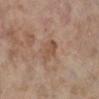- notes — total-body-photography surveillance lesion; no biopsy
- body site — the right lower leg
- image — total-body-photography crop, ~15 mm field of view
- subject — female, in their mid-80s or older
- size — ~3 mm (longest diameter)
- automated metrics — a lesion area of about 5 mm² and two-axis asymmetry of about 0.2; an automated nevus-likeness rating near 0 out of 100 and a detector confidence of about 100 out of 100 that the crop contains a lesion
- illumination — white-light